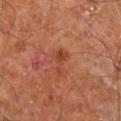Impression: Recorded during total-body skin imaging; not selected for excision or biopsy. Image and clinical context: The lesion is on the leg. A 15 mm close-up tile from a total-body photography series done for melanoma screening. A male patient aged approximately 60. Automated image analysis of the tile measured a border-irregularity rating of about 6/10 and peripheral color asymmetry of about 0.5.